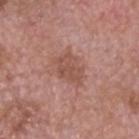Clinical impression: The lesion was tiled from a total-body skin photograph and was not biopsied. Clinical summary: A lesion tile, about 15 mm wide, cut from a 3D total-body photograph. The lesion is located on the head or neck. The patient is a male aged approximately 75. This is a white-light tile.